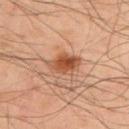Q: Is there a histopathology result?
A: no biopsy performed (imaged during a skin exam)
Q: What lighting was used for the tile?
A: cross-polarized
Q: What is the anatomic site?
A: the back
Q: What is the imaging modality?
A: ~15 mm tile from a whole-body skin photo
Q: What are the patient's age and sex?
A: male, aged around 50
Q: Lesion size?
A: ~4.5 mm (longest diameter)